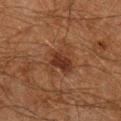<lesion>
  <biopsy_status>not biopsied; imaged during a skin examination</biopsy_status>
  <image>
    <source>total-body photography crop</source>
    <field_of_view_mm>15</field_of_view_mm>
  </image>
  <site>leg</site>
  <patient>
    <sex>male</sex>
    <age_approx>60</age_approx>
  </patient>
  <lesion_size>
    <long_diameter_mm_approx>4.5</long_diameter_mm_approx>
  </lesion_size>
  <lighting>cross-polarized</lighting>
</lesion>